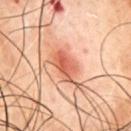Impression:
Part of a total-body skin-imaging series; this lesion was reviewed on a skin check and was not flagged for biopsy.
Background:
The patient is a male about 50 years old. The tile uses cross-polarized illumination. The lesion's longest dimension is about 3.5 mm. Located on the back. A lesion tile, about 15 mm wide, cut from a 3D total-body photograph.